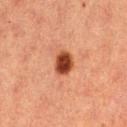Part of a total-body skin-imaging series; this lesion was reviewed on a skin check and was not flagged for biopsy. Automated tile analysis of the lesion measured a lesion area of about 6.5 mm², an outline eccentricity of about 0.55 (0 = round, 1 = elongated), and two-axis asymmetry of about 0.2. The analysis additionally found a mean CIELAB color near L≈39 a*≈26 b*≈32, a lesion–skin lightness drop of about 16, and a lesion-to-skin contrast of about 12.5 (normalized; higher = more distinct). And it measured border irregularity of about 1.5 on a 0–10 scale, a color-variation rating of about 3.5/10, and a peripheral color-asymmetry measure near 1. The software also gave a classifier nevus-likeness of about 100/100 and lesion-presence confidence of about 100/100. Imaged with cross-polarized lighting. The patient is a female aged 38 to 42. A close-up tile cropped from a whole-body skin photograph, about 15 mm across. Located on the right thigh.